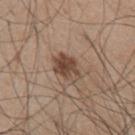Q: Was this lesion biopsied?
A: catalogued during a skin exam; not biopsied
Q: How large is the lesion?
A: about 4 mm
Q: Who is the patient?
A: male, approximately 45 years of age
Q: Lesion location?
A: the chest
Q: What lighting was used for the tile?
A: white-light illumination
Q: What kind of image is this?
A: ~15 mm crop, total-body skin-cancer survey